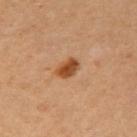No biopsy was performed on this lesion — it was imaged during a full skin examination and was not determined to be concerning.
An algorithmic analysis of the crop reported an average lesion color of about L≈49 a*≈25 b*≈38 (CIELAB), roughly 13 lightness units darker than nearby skin, and a lesion-to-skin contrast of about 10 (normalized; higher = more distinct). It also reported a detector confidence of about 100 out of 100 that the crop contains a lesion.
The patient is a female about 45 years old.
This is a cross-polarized tile.
A 15 mm crop from a total-body photograph taken for skin-cancer surveillance.
The lesion is on the right upper arm.